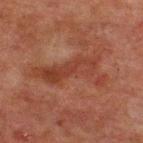| key | value |
|---|---|
| notes | total-body-photography surveillance lesion; no biopsy |
| lesion diameter | ≈8.5 mm |
| image | total-body-photography crop, ~15 mm field of view |
| location | the upper back |
| subject | male, aged 58 to 62 |
| lighting | cross-polarized |